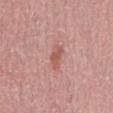biopsy status = total-body-photography surveillance lesion; no biopsy
image = ~15 mm tile from a whole-body skin photo
subject = male, aged 48 to 52
illumination = white-light illumination
site = the mid back